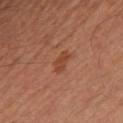Findings:
- notes · total-body-photography surveillance lesion; no biopsy
- location · the left leg
- acquisition · total-body-photography crop, ~15 mm field of view
- lesion size · about 2.5 mm
- subject · male, aged 48–52